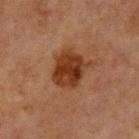biopsy_status: not biopsied; imaged during a skin examination
site: chest
patient:
  sex: male
  age_approx: 65
lighting: cross-polarized
lesion_size:
  long_diameter_mm_approx: 4.0
image:
  source: total-body photography crop
  field_of_view_mm: 15